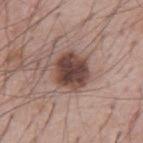Part of a total-body skin-imaging series; this lesion was reviewed on a skin check and was not flagged for biopsy.
A 15 mm crop from a total-body photograph taken for skin-cancer surveillance.
A male patient, about 55 years old.
Imaged with white-light lighting.
About 4.5 mm across.
Automated tile analysis of the lesion measured a lesion area of about 14 mm², an outline eccentricity of about 0.45 (0 = round, 1 = elongated), and a shape-asymmetry score of about 0.15 (0 = symmetric). The software also gave a mean CIELAB color near L≈44 a*≈18 b*≈22 and a normalized border contrast of about 11.5. And it measured a nevus-likeness score of about 60/100 and a detector confidence of about 100 out of 100 that the crop contains a lesion.